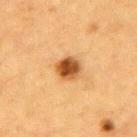A roughly 15 mm field-of-view crop from a total-body skin photograph.
This is a cross-polarized tile.
A male patient aged 83 or older.
Automated image analysis of the tile measured a lesion area of about 7 mm², an eccentricity of roughly 0.55, and two-axis asymmetry of about 0.15. The software also gave a mean CIELAB color near L≈48 a*≈23 b*≈40, a lesion–skin lightness drop of about 16, and a lesion-to-skin contrast of about 11 (normalized; higher = more distinct).
Longest diameter approximately 3 mm.
The lesion is located on the upper back.